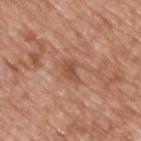This lesion was catalogued during total-body skin photography and was not selected for biopsy.
From the upper back.
The patient is a male approximately 50 years of age.
The total-body-photography lesion software estimated an average lesion color of about L≈51 a*≈23 b*≈31 (CIELAB), about 8 CIELAB-L* units darker than the surrounding skin, and a normalized lesion–skin contrast near 6.
Cropped from a total-body skin-imaging series; the visible field is about 15 mm.
Captured under white-light illumination.
Longest diameter approximately 2.5 mm.